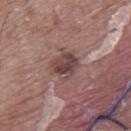  biopsy_status: not biopsied; imaged during a skin examination
  automated_metrics:
    border_irregularity_0_10: 2.0
    peripheral_color_asymmetry: 2.5
    nevus_likeness_0_100: 0
    lesion_detection_confidence_0_100: 100
  patient:
    sex: male
    age_approx: 70
  lesion_size:
    long_diameter_mm_approx: 4.0
  lighting: white-light
  site: mid back
  image:
    source: total-body photography crop
    field_of_view_mm: 15